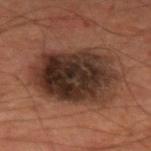Recorded during total-body skin imaging; not selected for excision or biopsy.
A male patient, aged 63–67.
Approximately 8.5 mm at its widest.
Cropped from a whole-body photographic skin survey; the tile spans about 15 mm.
On the left thigh.
Captured under cross-polarized illumination.